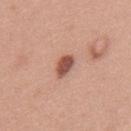{
  "biopsy_status": "not biopsied; imaged during a skin examination",
  "image": {
    "source": "total-body photography crop",
    "field_of_view_mm": 15
  },
  "patient": {
    "sex": "female",
    "age_approx": 40
  },
  "site": "upper back"
}